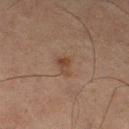No biopsy was performed on this lesion — it was imaged during a full skin examination and was not determined to be concerning. Approximately 2.5 mm at its widest. Captured under cross-polarized illumination. The lesion is on the left thigh. The lesion-visualizer software estimated an area of roughly 4 mm² and two-axis asymmetry of about 0.4. The analysis additionally found lesion-presence confidence of about 100/100. This image is a 15 mm lesion crop taken from a total-body photograph. The subject is a male about 65 years old.